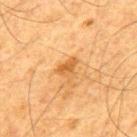notes=no biopsy performed (imaged during a skin exam)
subject=male, aged around 65
imaging modality=~15 mm tile from a whole-body skin photo
anatomic site=the mid back